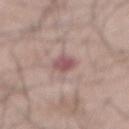Acquisition and patient details:
A male subject, aged 48–52. A 15 mm crop from a total-body photograph taken for skin-cancer surveillance. The lesion is located on the abdomen. The tile uses white-light illumination. About 2.5 mm across.